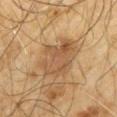No biopsy was performed on this lesion — it was imaged during a full skin examination and was not determined to be concerning. A 15 mm close-up extracted from a 3D total-body photography capture. The lesion's longest dimension is about 5.5 mm. Located on the back. This is a cross-polarized tile. The subject is a male aged approximately 65. An algorithmic analysis of the crop reported a border-irregularity rating of about 3/10 and a within-lesion color-variation index near 4.5/10.